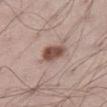Part of a total-body skin-imaging series; this lesion was reviewed on a skin check and was not flagged for biopsy. On the left thigh. Automated image analysis of the tile measured an area of roughly 6 mm² and a shape eccentricity near 0.8. The software also gave an average lesion color of about L≈49 a*≈19 b*≈24 (CIELAB) and about 15 CIELAB-L* units darker than the surrounding skin. The analysis additionally found a border-irregularity index near 2/10, a color-variation rating of about 3.5/10, and a peripheral color-asymmetry measure near 1. Imaged with white-light lighting. Cropped from a total-body skin-imaging series; the visible field is about 15 mm. The lesion's longest dimension is about 3.5 mm. A male subject aged 68–72.